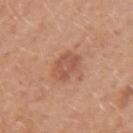Q: Was this lesion biopsied?
A: no biopsy performed (imaged during a skin exam)
Q: How large is the lesion?
A: about 3.5 mm
Q: Patient demographics?
A: female, aged around 40
Q: Illumination type?
A: white-light illumination
Q: What did automated image analysis measure?
A: an average lesion color of about L≈54 a*≈24 b*≈31 (CIELAB), a lesion–skin lightness drop of about 8, and a lesion-to-skin contrast of about 6 (normalized; higher = more distinct)
Q: Where on the body is the lesion?
A: the left upper arm
Q: What kind of image is this?
A: 15 mm crop, total-body photography